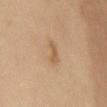This lesion was catalogued during total-body skin photography and was not selected for biopsy. A female subject aged around 60. From the chest. Automated image analysis of the tile measured a lesion color around L≈49 a*≈16 b*≈31 in CIELAB and a normalized border contrast of about 6. The tile uses cross-polarized illumination. A 15 mm crop from a total-body photograph taken for skin-cancer surveillance.